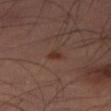  lesion_size:
    long_diameter_mm_approx: 1.5
  site: right thigh
  patient:
    sex: male
    age_approx: 50
  image:
    source: total-body photography crop
    field_of_view_mm: 15
  lighting: cross-polarized